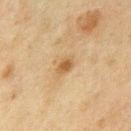<tbp_lesion>
<biopsy_status>not biopsied; imaged during a skin examination</biopsy_status>
<image>
  <source>total-body photography crop</source>
  <field_of_view_mm>15</field_of_view_mm>
</image>
<patient>
  <sex>male</sex>
  <age_approx>75</age_approx>
</patient>
<site>front of the torso</site>
<lesion_size>
  <long_diameter_mm_approx>2.5</long_diameter_mm_approx>
</lesion_size>
<automated_metrics>
  <area_mm2_approx>3.0</area_mm2_approx>
  <eccentricity>0.8</eccentricity>
  <shape_asymmetry>0.3</shape_asymmetry>
  <cielab_L>46</cielab_L>
  <cielab_a>15</cielab_a>
  <cielab_b>33</cielab_b>
  <vs_skin_darker_L>9.0</vs_skin_darker_L>
  <vs_skin_contrast_norm>7.5</vs_skin_contrast_norm>
  <nevus_likeness_0_100>75</nevus_likeness_0_100>
  <lesion_detection_confidence_0_100>100</lesion_detection_confidence_0_100>
</automated_metrics>
<lighting>cross-polarized</lighting>
</tbp_lesion>